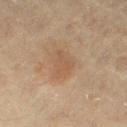Findings:
• biopsy status · total-body-photography surveillance lesion; no biopsy
• image · total-body-photography crop, ~15 mm field of view
• lesion diameter · ≈3 mm
• lighting · cross-polarized illumination
• patient · female, aged around 65
• body site · the leg
• automated metrics · a footprint of about 5 mm² and a shape eccentricity near 0.6; border irregularity of about 4.5 on a 0–10 scale, internal color variation of about 1 on a 0–10 scale, and radial color variation of about 0.5; an automated nevus-likeness rating near 60 out of 100 and a detector confidence of about 100 out of 100 that the crop contains a lesion